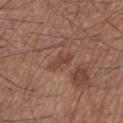Q: Was this lesion biopsied?
A: imaged on a skin check; not biopsied
Q: Where on the body is the lesion?
A: the left lower leg
Q: What kind of image is this?
A: ~15 mm tile from a whole-body skin photo
Q: Who is the patient?
A: male, approximately 60 years of age
Q: What lighting was used for the tile?
A: white-light
Q: What did automated image analysis measure?
A: a shape eccentricity near 0.85 and two-axis asymmetry of about 0.3